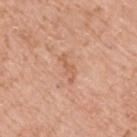Captured under white-light illumination.
On the upper back.
The patient is a male in their mid- to late 70s.
A 15 mm close-up tile from a total-body photography series done for melanoma screening.
Approximately 3.5 mm at its widest.